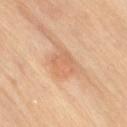Impression:
Captured during whole-body skin photography for melanoma surveillance; the lesion was not biopsied.
Context:
Automated image analysis of the tile measured an outline eccentricity of about 0.75 (0 = round, 1 = elongated) and two-axis asymmetry of about 0.3. It also reported a lesion–skin lightness drop of about 8 and a normalized lesion–skin contrast near 5. And it measured an automated nevus-likeness rating near 5 out of 100. The tile uses cross-polarized illumination. Located on the right thigh. Longest diameter approximately 4 mm. A region of skin cropped from a whole-body photographic capture, roughly 15 mm wide. The patient is a female roughly 70 years of age.